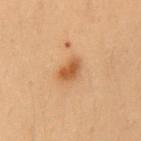biopsy_status: not biopsied; imaged during a skin examination
lesion_size:
  long_diameter_mm_approx: 3.0
site: mid back
patient:
  sex: female
  age_approx: 40
image:
  source: total-body photography crop
  field_of_view_mm: 15